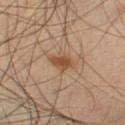Assessment: This lesion was catalogued during total-body skin photography and was not selected for biopsy. Context: Imaged with cross-polarized lighting. The lesion is located on the left thigh. A male patient, aged 38–42. An algorithmic analysis of the crop reported a lesion area of about 5 mm². The analysis additionally found a detector confidence of about 100 out of 100 that the crop contains a lesion. A close-up tile cropped from a whole-body skin photograph, about 15 mm across.